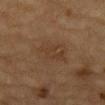{"biopsy_status": "not biopsied; imaged during a skin examination", "patient": {"sex": "male", "age_approx": "85+"}, "image": {"source": "total-body photography crop", "field_of_view_mm": 15}, "site": "mid back"}